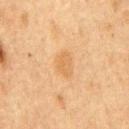Impression: This lesion was catalogued during total-body skin photography and was not selected for biopsy. Context: Located on the chest. Longest diameter approximately 3 mm. Captured under cross-polarized illumination. The subject is a male aged 73 to 77. A roughly 15 mm field-of-view crop from a total-body skin photograph.